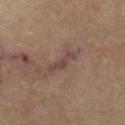Findings:
• notes: no biopsy performed (imaged during a skin exam)
• lesion size: ≈3.5 mm
• patient: female, roughly 65 years of age
• illumination: cross-polarized
• image: ~15 mm crop, total-body skin-cancer survey
• anatomic site: the left thigh